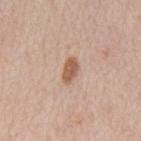biopsy status: catalogued during a skin exam; not biopsied | image: total-body-photography crop, ~15 mm field of view | lesion size: ~3 mm (longest diameter) | illumination: white-light illumination | location: the mid back | TBP lesion metrics: a footprint of about 4 mm²; an average lesion color of about L≈57 a*≈20 b*≈31 (CIELAB), a lesion–skin lightness drop of about 12, and a lesion-to-skin contrast of about 9 (normalized; higher = more distinct); a nevus-likeness score of about 90/100 and lesion-presence confidence of about 100/100 | patient: male, aged 63 to 67.